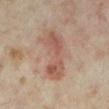<lesion>
  <lesion_size>
    <long_diameter_mm_approx>6.5</long_diameter_mm_approx>
  </lesion_size>
  <lighting>cross-polarized</lighting>
  <site>left lower leg</site>
  <automated_metrics>
    <cielab_L>52</cielab_L>
    <cielab_a>20</cielab_a>
    <cielab_b>26</cielab_b>
    <vs_skin_darker_L>9.0</vs_skin_darker_L>
    <vs_skin_contrast_norm>6.5</vs_skin_contrast_norm>
    <nevus_likeness_0_100>15</nevus_likeness_0_100>
    <lesion_detection_confidence_0_100>100</lesion_detection_confidence_0_100>
  </automated_metrics>
  <patient>
    <sex>female</sex>
    <age_approx>35</age_approx>
  </patient>
  <image>
    <source>total-body photography crop</source>
    <field_of_view_mm>15</field_of_view_mm>
  </image>
</lesion>